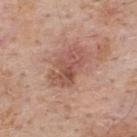The recorded lesion diameter is about 5 mm.
Captured under white-light illumination.
A 15 mm crop from a total-body photograph taken for skin-cancer surveillance.
The lesion is located on the upper back.
The patient is a male in their 60s.
Automated image analysis of the tile measured an average lesion color of about L≈53 a*≈23 b*≈27 (CIELAB), about 10 CIELAB-L* units darker than the surrounding skin, and a lesion-to-skin contrast of about 7 (normalized; higher = more distinct).